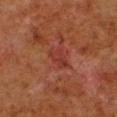{"biopsy_status": "not biopsied; imaged during a skin examination", "lighting": "cross-polarized", "lesion_size": {"long_diameter_mm_approx": 3.0}, "site": "left lower leg", "patient": {"sex": "male", "age_approx": 80}, "image": {"source": "total-body photography crop", "field_of_view_mm": 15}}